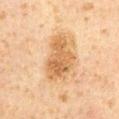biopsy status: imaged on a skin check; not biopsied | location: the chest | acquisition: 15 mm crop, total-body photography | tile lighting: cross-polarized | automated lesion analysis: a mean CIELAB color near L≈53 a*≈18 b*≈36, about 10 CIELAB-L* units darker than the surrounding skin, and a normalized lesion–skin contrast near 8; a classifier nevus-likeness of about 25/100 and a detector confidence of about 100 out of 100 that the crop contains a lesion | subject: male, aged around 60 | lesion diameter: ≈6 mm.